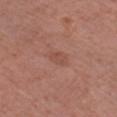| key | value |
|---|---|
| follow-up | no biopsy performed (imaged during a skin exam) |
| subject | male, aged approximately 60 |
| anatomic site | the left forearm |
| tile lighting | white-light illumination |
| size | ≈3 mm |
| imaging modality | 15 mm crop, total-body photography |